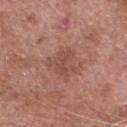Recorded during total-body skin imaging; not selected for excision or biopsy.
The lesion is on the head or neck.
The subject is a male aged 73 to 77.
Measured at roughly 3.5 mm in maximum diameter.
Cropped from a whole-body photographic skin survey; the tile spans about 15 mm.